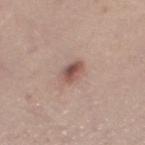Assessment:
No biopsy was performed on this lesion — it was imaged during a full skin examination and was not determined to be concerning.
Image and clinical context:
Captured under white-light illumination. The total-body-photography lesion software estimated an average lesion color of about L≈52 a*≈20 b*≈23 (CIELAB), roughly 12 lightness units darker than nearby skin, and a normalized border contrast of about 8.5. A female patient approximately 30 years of age. The lesion's longest dimension is about 3 mm. The lesion is on the left thigh. A 15 mm crop from a total-body photograph taken for skin-cancer surveillance.